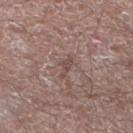biopsy status: no biopsy performed (imaged during a skin exam)
site: the left lower leg
lighting: white-light
patient: male, in their mid- to late 60s
image source: ~15 mm tile from a whole-body skin photo
lesion size: ~3 mm (longest diameter)